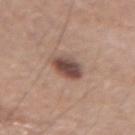{"biopsy_status": "not biopsied; imaged during a skin examination", "site": "left forearm", "patient": {"sex": "male", "age_approx": 60}, "lesion_size": {"long_diameter_mm_approx": 3.5}, "automated_metrics": {"eccentricity": 0.5, "shape_asymmetry": 0.15, "cielab_L": 47, "cielab_a": 18, "cielab_b": 23, "vs_skin_darker_L": 14.0, "vs_skin_contrast_norm": 10.5}, "image": {"source": "total-body photography crop", "field_of_view_mm": 15}, "lighting": "white-light"}